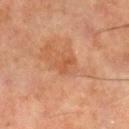Findings:
• notes — total-body-photography surveillance lesion; no biopsy
• automated lesion analysis — border irregularity of about 4.5 on a 0–10 scale and a peripheral color-asymmetry measure near 0; an automated nevus-likeness rating near 0 out of 100 and a detector confidence of about 100 out of 100 that the crop contains a lesion
• subject — male, aged around 60
• illumination — cross-polarized illumination
• anatomic site — the right lower leg
• lesion diameter — ≈2.5 mm
• imaging modality — ~15 mm tile from a whole-body skin photo